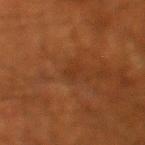The lesion was photographed on a routine skin check and not biopsied; there is no pathology result.
The lesion is located on the leg.
The subject is a male aged approximately 60.
This image is a 15 mm lesion crop taken from a total-body photograph.